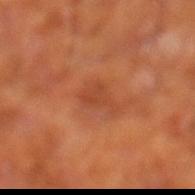Case summary:
* biopsy status — no biopsy performed (imaged during a skin exam)
* subject — male, about 65 years old
* location — the left lower leg
* diameter — ~3.5 mm (longest diameter)
* image — total-body-photography crop, ~15 mm field of view
* TBP lesion metrics — a footprint of about 5 mm², an outline eccentricity of about 0.8 (0 = round, 1 = elongated), and a symmetry-axis asymmetry near 0.5; border irregularity of about 5.5 on a 0–10 scale and a peripheral color-asymmetry measure near 0.5; an automated nevus-likeness rating near 0 out of 100 and a detector confidence of about 100 out of 100 that the crop contains a lesion